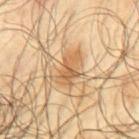A male subject aged approximately 65. Cropped from a whole-body photographic skin survey; the tile spans about 15 mm. The lesion is on the back.